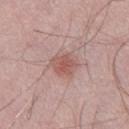Findings:
• notes · no biopsy performed (imaged during a skin exam)
• lesion diameter · ≈3 mm
• automated metrics · a lesion color around L≈55 a*≈23 b*≈24 in CIELAB, a lesion–skin lightness drop of about 9, and a normalized lesion–skin contrast near 7; a border-irregularity rating of about 2/10, a within-lesion color-variation index near 3/10, and a peripheral color-asymmetry measure near 1; a classifier nevus-likeness of about 75/100 and a detector confidence of about 100 out of 100 that the crop contains a lesion
• anatomic site · the right thigh
• patient · male, aged 53 to 57
• image source · ~15 mm tile from a whole-body skin photo